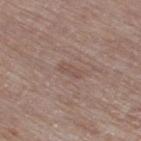workup: total-body-photography surveillance lesion; no biopsy | automated metrics: an eccentricity of roughly 0.9 and a shape-asymmetry score of about 0.35 (0 = symmetric); a border-irregularity rating of about 3.5/10, internal color variation of about 0 on a 0–10 scale, and peripheral color asymmetry of about 0; an automated nevus-likeness rating near 0 out of 100 and lesion-presence confidence of about 100/100 | patient: male, in their mid-80s or older | site: the right thigh | illumination: white-light illumination | size: ~2.5 mm (longest diameter) | image source: ~15 mm tile from a whole-body skin photo.